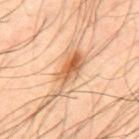{
  "image": {
    "source": "total-body photography crop",
    "field_of_view_mm": 15
  },
  "lighting": "cross-polarized",
  "site": "upper back",
  "lesion_size": {
    "long_diameter_mm_approx": 6.0
  },
  "patient": {
    "sex": "male",
    "age_approx": 50
  }
}